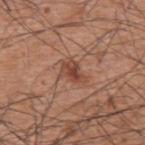follow-up: total-body-photography surveillance lesion; no biopsy | image: ~15 mm crop, total-body skin-cancer survey | subject: male, in their 60s | tile lighting: white-light | site: the upper back.